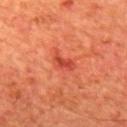Part of a total-body skin-imaging series; this lesion was reviewed on a skin check and was not flagged for biopsy. A 15 mm close-up tile from a total-body photography series done for melanoma screening. The lesion is located on the back. A male patient aged around 65. Measured at roughly 3 mm in maximum diameter. The tile uses cross-polarized illumination.